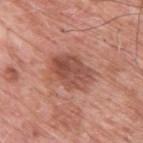Q: Was a biopsy performed?
A: no biopsy performed (imaged during a skin exam)
Q: What is the imaging modality?
A: ~15 mm crop, total-body skin-cancer survey
Q: Lesion size?
A: ≈5 mm
Q: What is the anatomic site?
A: the upper back
Q: Patient demographics?
A: male, roughly 60 years of age